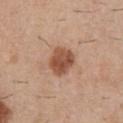The lesion was photographed on a routine skin check and not biopsied; there is no pathology result. The tile uses white-light illumination. Longest diameter approximately 3.5 mm. A male subject approximately 30 years of age. On the chest. A roughly 15 mm field-of-view crop from a total-body skin photograph.